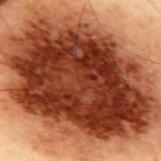| key | value |
|---|---|
| notes | imaged on a skin check; not biopsied |
| site | the upper back |
| patient | male, aged approximately 55 |
| imaging modality | ~15 mm crop, total-body skin-cancer survey |